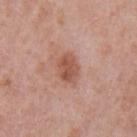Recorded during total-body skin imaging; not selected for excision or biopsy. The lesion-visualizer software estimated a lesion color around L≈53 a*≈24 b*≈29 in CIELAB, a lesion–skin lightness drop of about 11, and a normalized lesion–skin contrast near 7.5. It also reported a detector confidence of about 100 out of 100 that the crop contains a lesion. Approximately 3.5 mm at its widest. A 15 mm close-up tile from a total-body photography series done for melanoma screening. A male subject about 40 years old. From the left upper arm. Imaged with white-light lighting.